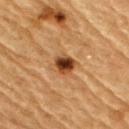• anatomic site · the right upper arm
• image-analysis metrics · a footprint of about 6.5 mm² and a symmetry-axis asymmetry near 0.25; an average lesion color of about L≈38 a*≈20 b*≈33 (CIELAB), a lesion–skin lightness drop of about 14, and a normalized border contrast of about 11.5; border irregularity of about 2.5 on a 0–10 scale, a within-lesion color-variation index near 7.5/10, and peripheral color asymmetry of about 2
• patient · male, approximately 85 years of age or older
• lesion diameter · ~3 mm (longest diameter)
• tile lighting · cross-polarized
• image source · ~15 mm tile from a whole-body skin photo